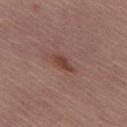The lesion's longest dimension is about 3 mm. The tile uses white-light illumination. From the left thigh. Cropped from a whole-body photographic skin survey; the tile spans about 15 mm. The subject is a female aged 63 to 67. The total-body-photography lesion software estimated an area of roughly 3 mm², an outline eccentricity of about 0.9 (0 = round, 1 = elongated), and two-axis asymmetry of about 0.35. And it measured a lesion color around L≈41 a*≈22 b*≈24 in CIELAB, about 9 CIELAB-L* units darker than the surrounding skin, and a normalized border contrast of about 8. The analysis additionally found a border-irregularity rating of about 3.5/10. The analysis additionally found an automated nevus-likeness rating near 40 out of 100 and lesion-presence confidence of about 100/100.